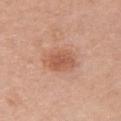Imaged during a routine full-body skin examination; the lesion was not biopsied and no histopathology is available. The lesion-visualizer software estimated an area of roughly 10 mm², an outline eccentricity of about 0.75 (0 = round, 1 = elongated), and two-axis asymmetry of about 0.15. It also reported a normalized border contrast of about 6.5. And it measured a nevus-likeness score of about 95/100 and a lesion-detection confidence of about 100/100. A female patient aged 58–62. A region of skin cropped from a whole-body photographic capture, roughly 15 mm wide. Located on the chest. The tile uses white-light illumination.